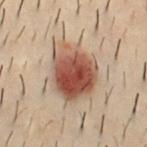* follow-up · catalogued during a skin exam; not biopsied
* site · the chest
* patient · male, about 30 years old
* imaging modality · ~15 mm crop, total-body skin-cancer survey
* TBP lesion metrics · an area of roughly 27 mm², an outline eccentricity of about 0.5 (0 = round, 1 = elongated), and a shape-asymmetry score of about 0.2 (0 = symmetric); a classifier nevus-likeness of about 100/100 and a lesion-detection confidence of about 100/100